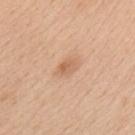Impression: The lesion was tiled from a total-body skin photograph and was not biopsied. Background: A 15 mm close-up extracted from a 3D total-body photography capture. The lesion is on the upper back. The subject is a male aged 58–62. The recorded lesion diameter is about 2.5 mm. Captured under white-light illumination.